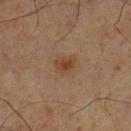The lesion was tiled from a total-body skin photograph and was not biopsied. Imaged with cross-polarized lighting. A close-up tile cropped from a whole-body skin photograph, about 15 mm across. The subject is a male aged around 65. Located on the right lower leg.